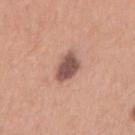Impression: Imaged during a routine full-body skin examination; the lesion was not biopsied and no histopathology is available. Background: A male patient, approximately 30 years of age. The lesion is located on the right upper arm. A lesion tile, about 15 mm wide, cut from a 3D total-body photograph. Imaged with white-light lighting.